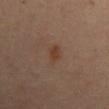workup = no biopsy performed (imaged during a skin exam)
lesion size = ≈3 mm
image = ~15 mm crop, total-body skin-cancer survey
automated metrics = a footprint of about 4 mm², an eccentricity of roughly 0.85, and two-axis asymmetry of about 0.3
body site = the mid back
patient = female, about 65 years old